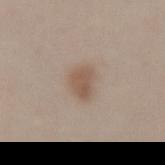Q: Was this lesion biopsied?
A: imaged on a skin check; not biopsied
Q: Patient demographics?
A: female, aged approximately 30
Q: How was the tile lit?
A: white-light
Q: Lesion location?
A: the lower back
Q: What kind of image is this?
A: ~15 mm crop, total-body skin-cancer survey